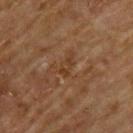Clinical impression: Imaged during a routine full-body skin examination; the lesion was not biopsied and no histopathology is available.